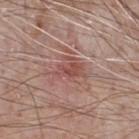Q: Was a biopsy performed?
A: total-body-photography surveillance lesion; no biopsy
Q: Lesion size?
A: ≈3.5 mm
Q: Who is the patient?
A: male, approximately 65 years of age
Q: Where on the body is the lesion?
A: the chest
Q: How was the tile lit?
A: white-light illumination
Q: What is the imaging modality?
A: 15 mm crop, total-body photography